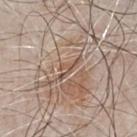{"biopsy_status": "not biopsied; imaged during a skin examination", "site": "chest", "patient": {"sex": "male", "age_approx": 65}, "lesion_size": {"long_diameter_mm_approx": 3.5}, "image": {"source": "total-body photography crop", "field_of_view_mm": 15}, "automated_metrics": {"border_irregularity_0_10": 5.5}}